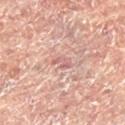Clinical impression: This lesion was catalogued during total-body skin photography and was not selected for biopsy. Acquisition and patient details: The lesion-visualizer software estimated a color-variation rating of about 0/10 and radial color variation of about 0. Imaged with cross-polarized lighting. A female subject, aged around 80. A lesion tile, about 15 mm wide, cut from a 3D total-body photograph. Located on the right leg. The lesion's longest dimension is about 3 mm.